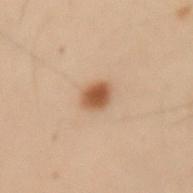| feature | finding |
|---|---|
| workup | total-body-photography surveillance lesion; no biopsy |
| acquisition | total-body-photography crop, ~15 mm field of view |
| patient | female, aged approximately 50 |
| site | the left forearm |